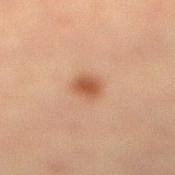workup = catalogued during a skin exam; not biopsied | acquisition = total-body-photography crop, ~15 mm field of view | lesion diameter = about 2.5 mm | illumination = cross-polarized illumination | patient = female, aged approximately 55 | body site = the right lower leg | image-analysis metrics = a lesion area of about 5.5 mm², an eccentricity of roughly 0.45, and two-axis asymmetry of about 0.2; a border-irregularity rating of about 2/10 and peripheral color asymmetry of about 1.